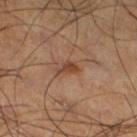This lesion was catalogued during total-body skin photography and was not selected for biopsy.
The tile uses cross-polarized illumination.
The lesion is located on the right thigh.
The patient is a male about 60 years old.
The total-body-photography lesion software estimated an area of roughly 3 mm², an outline eccentricity of about 0.85 (0 = round, 1 = elongated), and two-axis asymmetry of about 0.45. The analysis additionally found a border-irregularity index near 4.5/10 and peripheral color asymmetry of about 0.5.
This image is a 15 mm lesion crop taken from a total-body photograph.